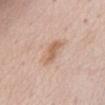notes=total-body-photography surveillance lesion; no biopsy | body site=the chest | patient=female, about 70 years old | image=total-body-photography crop, ~15 mm field of view | lesion diameter=≈4 mm.